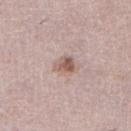Notes:
– biopsy status — catalogued during a skin exam; not biopsied
– size — ≈3 mm
– location — the left lower leg
– image source — total-body-photography crop, ~15 mm field of view
– illumination — white-light illumination
– subject — female, about 50 years old